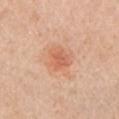* notes · catalogued during a skin exam; not biopsied
* size · about 3 mm
* patient · female, aged 68 to 72
* acquisition · ~15 mm crop, total-body skin-cancer survey
* body site · the left upper arm
* tile lighting · white-light illumination